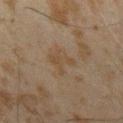patient:
  sex: male
  age_approx: 45
lesion_size:
  long_diameter_mm_approx: 3.0
site: arm
lighting: cross-polarized
image:
  source: total-body photography crop
  field_of_view_mm: 15